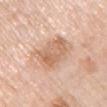Q: Was a biopsy performed?
A: catalogued during a skin exam; not biopsied
Q: Who is the patient?
A: female, roughly 65 years of age
Q: Automated lesion metrics?
A: a mean CIELAB color near L≈65 a*≈20 b*≈32, roughly 10 lightness units darker than nearby skin, and a normalized lesion–skin contrast near 6.5; border irregularity of about 3 on a 0–10 scale and radial color variation of about 1.5; an automated nevus-likeness rating near 0 out of 100 and a lesion-detection confidence of about 100/100
Q: Lesion location?
A: the left upper arm
Q: Illumination type?
A: white-light
Q: How large is the lesion?
A: ~4.5 mm (longest diameter)
Q: What kind of image is this?
A: ~15 mm tile from a whole-body skin photo